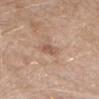Imaged with white-light lighting.
The subject is a female aged approximately 40.
The lesion is on the head or neck.
Automated image analysis of the tile measured a lesion area of about 3.5 mm², an eccentricity of roughly 0.7, and two-axis asymmetry of about 0.2. The software also gave a mean CIELAB color near L≈56 a*≈18 b*≈28 and about 9 CIELAB-L* units darker than the surrounding skin. It also reported a border-irregularity index near 2/10, internal color variation of about 1.5 on a 0–10 scale, and radial color variation of about 0.5.
A roughly 15 mm field-of-view crop from a total-body skin photograph.
Measured at roughly 2.5 mm in maximum diameter.